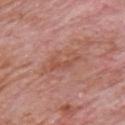| field | value |
|---|---|
| workup | catalogued during a skin exam; not biopsied |
| lesion diameter | ≈5.5 mm |
| illumination | white-light |
| patient | male, approximately 80 years of age |
| location | the front of the torso |
| imaging modality | total-body-photography crop, ~15 mm field of view |